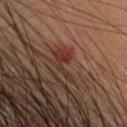Q: Is there a histopathology result?
A: imaged on a skin check; not biopsied
Q: Lesion size?
A: ≈4.5 mm
Q: What are the patient's age and sex?
A: female, roughly 45 years of age
Q: Where on the body is the lesion?
A: the head or neck
Q: What did automated image analysis measure?
A: a footprint of about 4.5 mm²; an average lesion color of about L≈33 a*≈22 b*≈22 (CIELAB) and a normalized border contrast of about 6.5
Q: What is the imaging modality?
A: total-body-photography crop, ~15 mm field of view